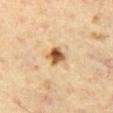Imaged during a routine full-body skin examination; the lesion was not biopsied and no histopathology is available.
A 15 mm close-up extracted from a 3D total-body photography capture.
A male patient, roughly 65 years of age.
From the leg.
The recorded lesion diameter is about 2.5 mm.